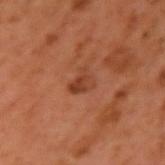Impression:
No biopsy was performed on this lesion — it was imaged during a full skin examination and was not determined to be concerning.
Background:
About 3 mm across. This is a cross-polarized tile. A male subject aged approximately 60. From the left upper arm. Automated tile analysis of the lesion measured an average lesion color of about L≈38 a*≈25 b*≈32 (CIELAB) and a lesion-to-skin contrast of about 7 (normalized; higher = more distinct). It also reported an automated nevus-likeness rating near 5 out of 100 and lesion-presence confidence of about 100/100. A 15 mm close-up extracted from a 3D total-body photography capture.